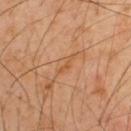| field | value |
|---|---|
| biopsy status | total-body-photography surveillance lesion; no biopsy |
| lighting | cross-polarized illumination |
| automated lesion analysis | an area of roughly 2.5 mm², an eccentricity of roughly 0.95, and a shape-asymmetry score of about 0.45 (0 = symmetric); an average lesion color of about L≈55 a*≈23 b*≈39 (CIELAB), about 6 CIELAB-L* units darker than the surrounding skin, and a lesion-to-skin contrast of about 5.5 (normalized; higher = more distinct) |
| acquisition | ~15 mm tile from a whole-body skin photo |
| anatomic site | the upper back |
| patient | male, in their 50s |
| size | ~3 mm (longest diameter) |